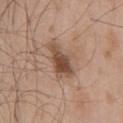Notes:
- notes — catalogued during a skin exam; not biopsied
- location — the front of the torso
- automated metrics — an average lesion color of about L≈49 a*≈18 b*≈29 (CIELAB) and a normalized lesion–skin contrast near 9; a nevus-likeness score of about 90/100 and a detector confidence of about 100 out of 100 that the crop contains a lesion
- subject — male, approximately 65 years of age
- diameter — ≈4 mm
- lighting — white-light illumination
- acquisition — total-body-photography crop, ~15 mm field of view